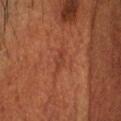Assessment: No biopsy was performed on this lesion — it was imaged during a full skin examination and was not determined to be concerning. Image and clinical context: Located on the head or neck. Measured at roughly 3 mm in maximum diameter. A 15 mm crop from a total-body photograph taken for skin-cancer surveillance. Automated tile analysis of the lesion measured about 5 CIELAB-L* units darker than the surrounding skin and a normalized lesion–skin contrast near 5. A male subject aged around 60. This is a cross-polarized tile.